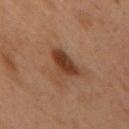workup — no biopsy performed (imaged during a skin exam)
image — 15 mm crop, total-body photography
tile lighting — cross-polarized
location — the left arm
patient — female, in their mid- to late 50s
diameter — about 4 mm